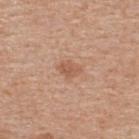<lesion>
  <biopsy_status>not biopsied; imaged during a skin examination</biopsy_status>
  <patient>
    <sex>male</sex>
    <age_approx>55</age_approx>
  </patient>
  <site>upper back</site>
  <image>
    <source>total-body photography crop</source>
    <field_of_view_mm>15</field_of_view_mm>
  </image>
</lesion>